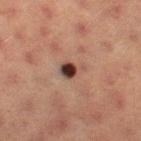<tbp_lesion>
  <biopsy_status>not biopsied; imaged during a skin examination</biopsy_status>
  <site>left thigh</site>
  <patient>
    <sex>female</sex>
    <age_approx>55</age_approx>
  </patient>
  <image>
    <source>total-body photography crop</source>
    <field_of_view_mm>15</field_of_view_mm>
  </image>
</tbp_lesion>